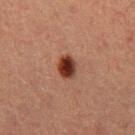{"biopsy_status": "not biopsied; imaged during a skin examination", "patient": {"sex": "female", "age_approx": 40}, "lesion_size": {"long_diameter_mm_approx": 3.0}, "lighting": "cross-polarized", "image": {"source": "total-body photography crop", "field_of_view_mm": 15}, "site": "right thigh"}